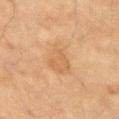workup: imaged on a skin check; not biopsied
site: the left upper arm
patient: male, aged 63–67
TBP lesion metrics: a footprint of about 11 mm², an outline eccentricity of about 0.85 (0 = round, 1 = elongated), and two-axis asymmetry of about 0.35; a mean CIELAB color near L≈50 a*≈17 b*≈32, about 5 CIELAB-L* units darker than the surrounding skin, and a lesion-to-skin contrast of about 4.5 (normalized; higher = more distinct); an automated nevus-likeness rating near 0 out of 100 and lesion-presence confidence of about 100/100
illumination: cross-polarized illumination
size: about 5 mm
imaging modality: 15 mm crop, total-body photography